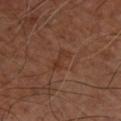Imaged during a routine full-body skin examination; the lesion was not biopsied and no histopathology is available. Longest diameter approximately 3 mm. This is a cross-polarized tile. A 15 mm crop from a total-body photograph taken for skin-cancer surveillance. A patient in their mid-60s. Automated image analysis of the tile measured a footprint of about 4 mm² and two-axis asymmetry of about 0.3. And it measured an average lesion color of about L≈34 a*≈21 b*≈27 (CIELAB), about 5 CIELAB-L* units darker than the surrounding skin, and a normalized border contrast of about 5.5. It also reported a border-irregularity index near 4/10, a within-lesion color-variation index near 2.5/10, and a peripheral color-asymmetry measure near 1. And it measured a nevus-likeness score of about 0/100 and lesion-presence confidence of about 100/100. On the left forearm.